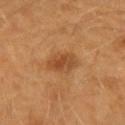notes — total-body-photography surveillance lesion; no biopsy
diameter — ≈3.5 mm
body site — the left upper arm
automated lesion analysis — an area of roughly 7 mm², an eccentricity of roughly 0.65, and a shape-asymmetry score of about 0.2 (0 = symmetric); border irregularity of about 2 on a 0–10 scale, a color-variation rating of about 5.5/10, and radial color variation of about 2; a classifier nevus-likeness of about 75/100 and lesion-presence confidence of about 100/100
patient — male, in their 40s
image — total-body-photography crop, ~15 mm field of view
illumination — cross-polarized